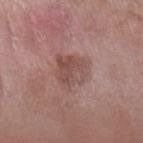{
  "site": "left forearm",
  "image": {
    "source": "total-body photography crop",
    "field_of_view_mm": 15
  },
  "patient": {
    "sex": "female",
    "age_approx": 75
  },
  "lesion_size": {
    "long_diameter_mm_approx": 4.0
  }
}